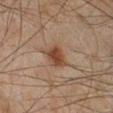Recorded during total-body skin imaging; not selected for excision or biopsy. The lesion's longest dimension is about 2.5 mm. Imaged with cross-polarized lighting. The lesion-visualizer software estimated a mean CIELAB color near L≈35 a*≈17 b*≈25, a lesion–skin lightness drop of about 9, and a normalized lesion–skin contrast near 8.5. A male patient, aged 43 to 47. A 15 mm crop from a total-body photograph taken for skin-cancer surveillance. The lesion is on the leg.